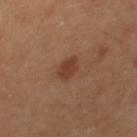  biopsy_status: not biopsied; imaged during a skin examination
  patient:
    sex: female
    age_approx: 60
  image:
    source: total-body photography crop
    field_of_view_mm: 15
  lesion_size:
    long_diameter_mm_approx: 2.5
  site: right thigh